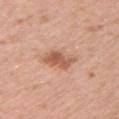This lesion was catalogued during total-body skin photography and was not selected for biopsy.
The lesion is located on the left upper arm.
A female subject, aged 38–42.
Captured under white-light illumination.
Cropped from a total-body skin-imaging series; the visible field is about 15 mm.
The lesion's longest dimension is about 3.5 mm.